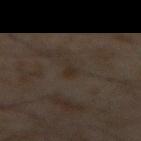notes: catalogued during a skin exam; not biopsied | TBP lesion metrics: a footprint of about 2.5 mm², an outline eccentricity of about 0.85 (0 = round, 1 = elongated), and a symmetry-axis asymmetry near 0.35; an average lesion color of about L≈23 a*≈9 b*≈18 (CIELAB), a lesion–skin lightness drop of about 4, and a normalized lesion–skin contrast near 6; a border-irregularity index near 4/10 and internal color variation of about 0.5 on a 0–10 scale | location: the abdomen | lesion diameter: ~2.5 mm (longest diameter) | subject: male, aged around 60 | acquisition: 15 mm crop, total-body photography.